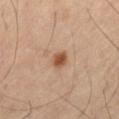No biopsy was performed on this lesion — it was imaged during a full skin examination and was not determined to be concerning. A 15 mm close-up tile from a total-body photography series done for melanoma screening. A male patient roughly 60 years of age. This is a cross-polarized tile. The lesion's longest dimension is about 2 mm. On the front of the torso.